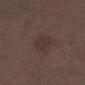Captured during whole-body skin photography for melanoma surveillance; the lesion was not biopsied.
Imaged with white-light lighting.
Longest diameter approximately 3 mm.
Cropped from a whole-body photographic skin survey; the tile spans about 15 mm.
An algorithmic analysis of the crop reported a lesion color around L≈32 a*≈14 b*≈20 in CIELAB and a lesion–skin lightness drop of about 5. And it measured a within-lesion color-variation index near 1.5/10 and a peripheral color-asymmetry measure near 0.5. The software also gave an automated nevus-likeness rating near 0 out of 100 and lesion-presence confidence of about 100/100.
On the right thigh.
A male patient aged 68 to 72.